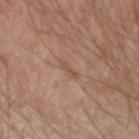{"biopsy_status": "not biopsied; imaged during a skin examination", "site": "left forearm", "patient": {"sex": "male", "age_approx": 50}, "lesion_size": {"long_diameter_mm_approx": 2.5}, "image": {"source": "total-body photography crop", "field_of_view_mm": 15}}